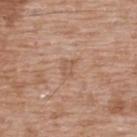Assessment: Recorded during total-body skin imaging; not selected for excision or biopsy. Image and clinical context: The tile uses white-light illumination. Cropped from a total-body skin-imaging series; the visible field is about 15 mm. A male subject, aged 48–52. The lesion is on the upper back. The total-body-photography lesion software estimated a classifier nevus-likeness of about 0/100.